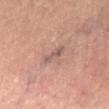Q: Is there a histopathology result?
A: imaged on a skin check; not biopsied
Q: What is the lesion's diameter?
A: ~3.5 mm (longest diameter)
Q: What is the anatomic site?
A: the leg
Q: What kind of image is this?
A: 15 mm crop, total-body photography
Q: Patient demographics?
A: male, aged 63 to 67
Q: How was the tile lit?
A: cross-polarized illumination